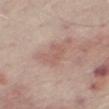Assessment: This lesion was catalogued during total-body skin photography and was not selected for biopsy. Image and clinical context: On the right thigh. The subject is a male in their mid- to late 60s. An algorithmic analysis of the crop reported a lesion area of about 6 mm² and an outline eccentricity of about 0.8 (0 = round, 1 = elongated). The software also gave a lesion color around L≈58 a*≈20 b*≈25 in CIELAB and a normalized lesion–skin contrast near 4.5. It also reported a border-irregularity index near 4.5/10, a color-variation rating of about 1.5/10, and radial color variation of about 0.5. It also reported an automated nevus-likeness rating near 0 out of 100 and a lesion-detection confidence of about 100/100. Captured under white-light illumination. A close-up tile cropped from a whole-body skin photograph, about 15 mm across.